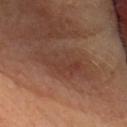{
  "biopsy_status": "not biopsied; imaged during a skin examination",
  "image": {
    "source": "total-body photography crop",
    "field_of_view_mm": 15
  },
  "automated_metrics": {
    "cielab_L": 40,
    "cielab_a": 21,
    "cielab_b": 27,
    "vs_skin_contrast_norm": 6.5,
    "nevus_likeness_0_100": 0,
    "lesion_detection_confidence_0_100": 90
  },
  "site": "head or neck",
  "patient": {
    "sex": "female",
    "age_approx": 70
  }
}